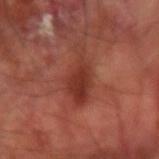biopsy status: imaged on a skin check; not biopsied
image source: 15 mm crop, total-body photography
body site: the arm
patient: male, about 60 years old